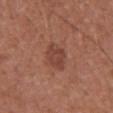Imaged during a routine full-body skin examination; the lesion was not biopsied and no histopathology is available.
Cropped from a total-body skin-imaging series; the visible field is about 15 mm.
A male subject, about 65 years old.
The lesion is on the abdomen.
Automated image analysis of the tile measured a footprint of about 7.5 mm² and an eccentricity of roughly 0.75. The software also gave a mean CIELAB color near L≈43 a*≈24 b*≈26 and roughly 8 lightness units darker than nearby skin. It also reported an automated nevus-likeness rating near 30 out of 100 and lesion-presence confidence of about 100/100.
The tile uses white-light illumination.